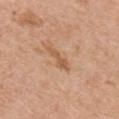Assessment: No biopsy was performed on this lesion — it was imaged during a full skin examination and was not determined to be concerning.